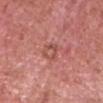A male patient aged approximately 50. A 15 mm close-up tile from a total-body photography series done for melanoma screening. The lesion's longest dimension is about 2.5 mm. The lesion is located on the head or neck.